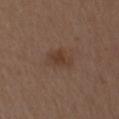Q: Was this lesion biopsied?
A: imaged on a skin check; not biopsied
Q: What are the patient's age and sex?
A: male, in their mid- to late 70s
Q: What kind of image is this?
A: total-body-photography crop, ~15 mm field of view
Q: What lighting was used for the tile?
A: white-light
Q: What is the lesion's diameter?
A: ~3.5 mm (longest diameter)
Q: What is the anatomic site?
A: the arm
Q: What did automated image analysis measure?
A: a lesion area of about 7 mm², a shape eccentricity near 0.75, and a symmetry-axis asymmetry near 0.3; a mean CIELAB color near L≈38 a*≈17 b*≈26, roughly 6 lightness units darker than nearby skin, and a normalized border contrast of about 6; border irregularity of about 3 on a 0–10 scale, a within-lesion color-variation index near 3/10, and radial color variation of about 1; a nevus-likeness score of about 30/100 and a lesion-detection confidence of about 100/100